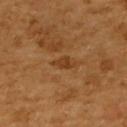The recorded lesion diameter is about 3 mm.
From the back.
A female patient roughly 55 years of age.
Captured under cross-polarized illumination.
A 15 mm close-up extracted from a 3D total-body photography capture.